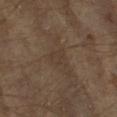Recorded during total-body skin imaging; not selected for excision or biopsy.
A male subject, in their mid-60s.
A lesion tile, about 15 mm wide, cut from a 3D total-body photograph.
The total-body-photography lesion software estimated a mean CIELAB color near L≈34 a*≈12 b*≈22, roughly 4 lightness units darker than nearby skin, and a lesion-to-skin contrast of about 4.5 (normalized; higher = more distinct). It also reported border irregularity of about 2.5 on a 0–10 scale and a within-lesion color-variation index near 1.5/10.
Longest diameter approximately 2.5 mm.
Imaged with cross-polarized lighting.
The lesion is on the right forearm.